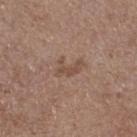The lesion was tiled from a total-body skin photograph and was not biopsied. Imaged with white-light lighting. A female subject aged 63–67. A roughly 15 mm field-of-view crop from a total-body skin photograph. About 3 mm across. Automated image analysis of the tile measured a within-lesion color-variation index near 0/10 and peripheral color asymmetry of about 0. The analysis additionally found a lesion-detection confidence of about 100/100. The lesion is on the right thigh.